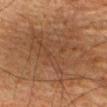Notes:
* follow-up — no biopsy performed (imaged during a skin exam)
* acquisition — ~15 mm crop, total-body skin-cancer survey
* location — the front of the torso
* lesion diameter — ≈14.5 mm
* illumination — cross-polarized
* subject — male, aged 78–82